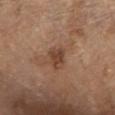No biopsy was performed on this lesion — it was imaged during a full skin examination and was not determined to be concerning. The patient is a male in their 70s. On the chest. A close-up tile cropped from a whole-body skin photograph, about 15 mm across. Imaged with white-light lighting. The lesion-visualizer software estimated a mean CIELAB color near L≈43 a*≈20 b*≈29, a lesion–skin lightness drop of about 10, and a lesion-to-skin contrast of about 7.5 (normalized; higher = more distinct). The analysis additionally found a border-irregularity index near 2.5/10 and internal color variation of about 2.5 on a 0–10 scale. Approximately 3 mm at its widest.